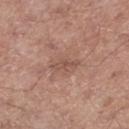workup: total-body-photography surveillance lesion; no biopsy | illumination: white-light illumination | size: about 3 mm | image: ~15 mm crop, total-body skin-cancer survey | location: the left lower leg | subject: male, aged 63–67.